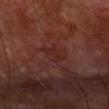Notes:
* site: the right forearm
* acquisition: total-body-photography crop, ~15 mm field of view
* lesion size: ≈2.5 mm
* tile lighting: cross-polarized illumination
* subject: male, aged 68 to 72
* automated metrics: an area of roughly 3 mm², an outline eccentricity of about 0.85 (0 = round, 1 = elongated), and a shape-asymmetry score of about 0.3 (0 = symmetric); a normalized lesion–skin contrast near 5; a border-irregularity rating of about 4/10 and internal color variation of about 0 on a 0–10 scale